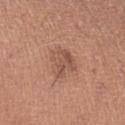{"biopsy_status": "not biopsied; imaged during a skin examination", "site": "left upper arm", "patient": {"sex": "female", "age_approx": 25}, "automated_metrics": {"area_mm2_approx": 9.0, "shape_asymmetry": 0.3, "cielab_L": 53, "cielab_a": 21, "cielab_b": 29, "vs_skin_darker_L": 8.0, "vs_skin_contrast_norm": 6.0, "border_irregularity_0_10": 3.5, "color_variation_0_10": 3.5, "peripheral_color_asymmetry": 1.5, "nevus_likeness_0_100": 20}, "lesion_size": {"long_diameter_mm_approx": 3.5}, "lighting": "white-light", "image": {"source": "total-body photography crop", "field_of_view_mm": 15}}